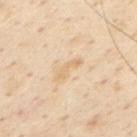Background:
The tile uses cross-polarized illumination. The lesion-visualizer software estimated an area of roughly 2.5 mm² and an eccentricity of roughly 0.95. It also reported an automated nevus-likeness rating near 0 out of 100. Cropped from a whole-body photographic skin survey; the tile spans about 15 mm. The lesion is located on the chest. About 3 mm across. A male patient approximately 55 years of age.